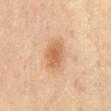Assessment: Part of a total-body skin-imaging series; this lesion was reviewed on a skin check and was not flagged for biopsy. Background: Longest diameter approximately 4 mm. From the front of the torso. This is a cross-polarized tile. A female patient aged approximately 65. This image is a 15 mm lesion crop taken from a total-body photograph.